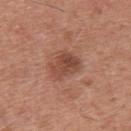Part of a total-body skin-imaging series; this lesion was reviewed on a skin check and was not flagged for biopsy. Automated tile analysis of the lesion measured a lesion area of about 8.5 mm², a shape eccentricity near 0.7, and two-axis asymmetry of about 0.25. It also reported a lesion color around L≈47 a*≈23 b*≈29 in CIELAB, about 10 CIELAB-L* units darker than the surrounding skin, and a normalized border contrast of about 7.5. A 15 mm close-up tile from a total-body photography series done for melanoma screening. A male subject roughly 30 years of age. The recorded lesion diameter is about 4 mm. On the upper back.